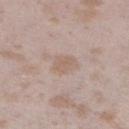Q: Who is the patient?
A: female, in their mid-20s
Q: Automated lesion metrics?
A: a border-irregularity index near 1.5/10, internal color variation of about 1.5 on a 0–10 scale, and a peripheral color-asymmetry measure near 0.5; a detector confidence of about 100 out of 100 that the crop contains a lesion
Q: What is the imaging modality?
A: ~15 mm tile from a whole-body skin photo
Q: Where on the body is the lesion?
A: the left lower leg
Q: How large is the lesion?
A: ~3 mm (longest diameter)
Q: How was the tile lit?
A: white-light